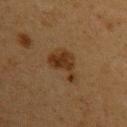{"biopsy_status": "not biopsied; imaged during a skin examination", "lesion_size": {"long_diameter_mm_approx": 4.5}, "site": "left upper arm", "image": {"source": "total-body photography crop", "field_of_view_mm": 15}, "patient": {"sex": "female", "age_approx": 40}, "automated_metrics": {"border_irregularity_0_10": 5.5, "color_variation_0_10": 3.5, "peripheral_color_asymmetry": 1.5}, "lighting": "cross-polarized"}